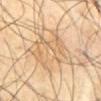• biopsy status — catalogued during a skin exam; not biopsied
• image-analysis metrics — a shape eccentricity near 0.7 and a symmetry-axis asymmetry near 0.3; a lesion color around L≈67 a*≈15 b*≈36 in CIELAB, roughly 8 lightness units darker than nearby skin, and a lesion-to-skin contrast of about 5 (normalized; higher = more distinct)
• lighting — cross-polarized
• subject — male, approximately 60 years of age
• lesion size — ≈7 mm
• anatomic site — the abdomen
• imaging modality — ~15 mm crop, total-body skin-cancer survey